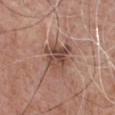Q: Was a biopsy performed?
A: no biopsy performed (imaged during a skin exam)
Q: What kind of image is this?
A: total-body-photography crop, ~15 mm field of view
Q: Lesion location?
A: the chest
Q: What is the lesion's diameter?
A: about 3.5 mm
Q: What are the patient's age and sex?
A: male, aged around 65
Q: What lighting was used for the tile?
A: white-light